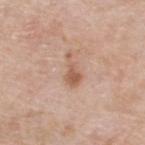The lesion was tiled from a total-body skin photograph and was not biopsied.
Automated tile analysis of the lesion measured a lesion area of about 5 mm², an eccentricity of roughly 0.9, and a symmetry-axis asymmetry near 0.55. The software also gave a mean CIELAB color near L≈58 a*≈20 b*≈30. And it measured border irregularity of about 5.5 on a 0–10 scale, internal color variation of about 2 on a 0–10 scale, and peripheral color asymmetry of about 0.5. It also reported a classifier nevus-likeness of about 30/100.
On the upper back.
A male patient, aged 58 to 62.
Approximately 4 mm at its widest.
A 15 mm close-up extracted from a 3D total-body photography capture.
This is a white-light tile.